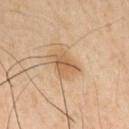Assessment:
Imaged during a routine full-body skin examination; the lesion was not biopsied and no histopathology is available.
Context:
A male subject roughly 40 years of age. An algorithmic analysis of the crop reported a nevus-likeness score of about 45/100 and a lesion-detection confidence of about 100/100. Captured under cross-polarized illumination. A 15 mm close-up tile from a total-body photography series done for melanoma screening. On the right upper arm.